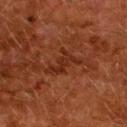Impression: Recorded during total-body skin imaging; not selected for excision or biopsy. Acquisition and patient details: Automated image analysis of the tile measured an average lesion color of about L≈24 a*≈22 b*≈27 (CIELAB), roughly 6 lightness units darker than nearby skin, and a normalized lesion–skin contrast near 6.5. And it measured border irregularity of about 9 on a 0–10 scale, a within-lesion color-variation index near 0.5/10, and peripheral color asymmetry of about 0. And it measured a nevus-likeness score of about 0/100. On the upper back. A 15 mm close-up extracted from a 3D total-body photography capture. This is a cross-polarized tile. A female patient, in their 50s.